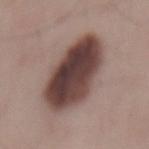The lesion was tiled from a total-body skin photograph and was not biopsied. An algorithmic analysis of the crop reported a border-irregularity index near 2/10, a color-variation rating of about 8/10, and a peripheral color-asymmetry measure near 3. A male subject, in their mid-50s. Cropped from a whole-body photographic skin survey; the tile spans about 15 mm. The lesion is located on the mid back.